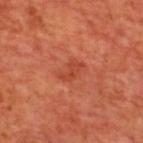Notes:
- workup: catalogued during a skin exam; not biopsied
- anatomic site: the upper back
- illumination: cross-polarized illumination
- patient: male, aged approximately 70
- size: about 3 mm
- image: ~15 mm tile from a whole-body skin photo